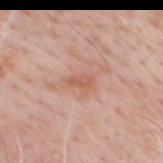Q: Is there a histopathology result?
A: imaged on a skin check; not biopsied
Q: How large is the lesion?
A: ≈2.5 mm
Q: What kind of image is this?
A: ~15 mm crop, total-body skin-cancer survey
Q: Automated lesion metrics?
A: a lesion color around L≈59 a*≈23 b*≈30 in CIELAB and roughly 8 lightness units darker than nearby skin; border irregularity of about 2.5 on a 0–10 scale, internal color variation of about 1.5 on a 0–10 scale, and a peripheral color-asymmetry measure near 0.5; a nevus-likeness score of about 0/100 and a lesion-detection confidence of about 100/100
Q: What lighting was used for the tile?
A: white-light illumination
Q: What is the anatomic site?
A: the chest
Q: Patient demographics?
A: male, aged 78 to 82